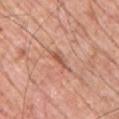The lesion was tiled from a total-body skin photograph and was not biopsied. From the front of the torso. A male patient, about 60 years old. Approximately 3 mm at its widest. Automated tile analysis of the lesion measured a border-irregularity index near 4/10, a within-lesion color-variation index near 1/10, and peripheral color asymmetry of about 0. It also reported a classifier nevus-likeness of about 0/100 and a lesion-detection confidence of about 70/100. This image is a 15 mm lesion crop taken from a total-body photograph.